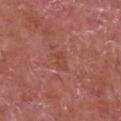Impression: The lesion was tiled from a total-body skin photograph and was not biopsied. Context: This image is a 15 mm lesion crop taken from a total-body photograph. A male patient aged 63 to 67. The lesion's longest dimension is about 2.5 mm. Captured under white-light illumination. From the chest. Automated tile analysis of the lesion measured a lesion area of about 3.5 mm² and a shape eccentricity near 0.8. The software also gave an average lesion color of about L≈46 a*≈28 b*≈29 (CIELAB) and roughly 5 lightness units darker than nearby skin. The analysis additionally found border irregularity of about 3 on a 0–10 scale, internal color variation of about 2 on a 0–10 scale, and peripheral color asymmetry of about 0.5.